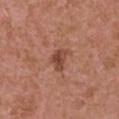The lesion was photographed on a routine skin check and not biopsied; there is no pathology result. Located on the arm. Automated image analysis of the tile measured a lesion area of about 5 mm² and a shape-asymmetry score of about 0.4 (0 = symmetric). It also reported an average lesion color of about L≈46 a*≈24 b*≈29 (CIELAB) and a normalized border contrast of about 7.5. The analysis additionally found a border-irregularity rating of about 4/10, a color-variation rating of about 3/10, and peripheral color asymmetry of about 1. A male subject aged around 75. The tile uses white-light illumination. A 15 mm close-up tile from a total-body photography series done for melanoma screening. The lesion's longest dimension is about 3 mm.